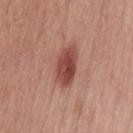Background:
The total-body-photography lesion software estimated a shape eccentricity near 0.85 and two-axis asymmetry of about 0.2. The analysis additionally found a within-lesion color-variation index near 4.5/10 and a peripheral color-asymmetry measure near 1.5. The analysis additionally found a classifier nevus-likeness of about 95/100 and a lesion-detection confidence of about 100/100. About 5 mm across. The tile uses white-light illumination. The lesion is on the lower back. A 15 mm close-up extracted from a 3D total-body photography capture. The patient is a female approximately 40 years of age.